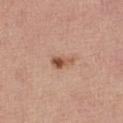Assessment:
Part of a total-body skin-imaging series; this lesion was reviewed on a skin check and was not flagged for biopsy.
Acquisition and patient details:
A 15 mm close-up extracted from a 3D total-body photography capture. On the leg. The lesion-visualizer software estimated a footprint of about 4 mm². And it measured a border-irregularity index near 3/10, a color-variation rating of about 4/10, and a peripheral color-asymmetry measure near 1.5. The software also gave an automated nevus-likeness rating near 85 out of 100. Measured at roughly 3 mm in maximum diameter. Captured under white-light illumination. The subject is a female aged 63 to 67.